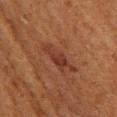Assessment:
Recorded during total-body skin imaging; not selected for excision or biopsy.
Image and clinical context:
A 15 mm crop from a total-body photograph taken for skin-cancer surveillance. A female patient, roughly 50 years of age. Located on the left thigh.